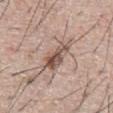biopsy status: no biopsy performed (imaged during a skin exam)
image source: ~15 mm crop, total-body skin-cancer survey
site: the abdomen
patient: male, aged 63–67